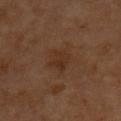The lesion was tiled from a total-body skin photograph and was not biopsied.
The lesion's longest dimension is about 2.5 mm.
Captured under cross-polarized illumination.
A close-up tile cropped from a whole-body skin photograph, about 15 mm across.
The lesion-visualizer software estimated a lesion area of about 5 mm², an outline eccentricity of about 0.55 (0 = round, 1 = elongated), and two-axis asymmetry of about 0.35. The software also gave a mean CIELAB color near L≈27 a*≈17 b*≈26, about 5 CIELAB-L* units darker than the surrounding skin, and a normalized lesion–skin contrast near 6. It also reported a border-irregularity index near 3/10, internal color variation of about 2 on a 0–10 scale, and peripheral color asymmetry of about 0.5.
The patient is a male aged approximately 60.
The lesion is on the chest.